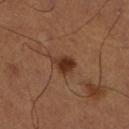Impression: Imaged during a routine full-body skin examination; the lesion was not biopsied and no histopathology is available. Context: Located on the leg. A male subject aged 48–52. A 15 mm close-up tile from a total-body photography series done for melanoma screening. The lesion-visualizer software estimated a footprint of about 5.5 mm², a shape eccentricity near 0.65, and a shape-asymmetry score of about 0.35 (0 = symmetric). The analysis additionally found roughly 11 lightness units darker than nearby skin and a normalized border contrast of about 9.5.